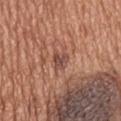Q: Was a biopsy performed?
A: catalogued during a skin exam; not biopsied
Q: Where on the body is the lesion?
A: the mid back
Q: Lesion size?
A: ≈2.5 mm
Q: Automated lesion metrics?
A: a footprint of about 4 mm², a shape eccentricity near 0.7, and two-axis asymmetry of about 0.35; a classifier nevus-likeness of about 0/100 and a detector confidence of about 100 out of 100 that the crop contains a lesion
Q: What is the imaging modality?
A: 15 mm crop, total-body photography
Q: Illumination type?
A: white-light illumination
Q: Who is the patient?
A: male, aged approximately 65